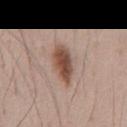Part of a total-body skin-imaging series; this lesion was reviewed on a skin check and was not flagged for biopsy. About 5.5 mm across. The total-body-photography lesion software estimated a footprint of about 9 mm², an outline eccentricity of about 0.9 (0 = round, 1 = elongated), and a shape-asymmetry score of about 0.25 (0 = symmetric). The analysis additionally found a mean CIELAB color near L≈49 a*≈19 b*≈26 and a lesion–skin lightness drop of about 14. It also reported border irregularity of about 3 on a 0–10 scale, a color-variation rating of about 4/10, and radial color variation of about 1. It also reported a lesion-detection confidence of about 100/100. A male subject aged approximately 45. On the abdomen. Cropped from a whole-body photographic skin survey; the tile spans about 15 mm.